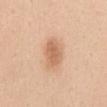Imaged during a routine full-body skin examination; the lesion was not biopsied and no histopathology is available. The subject is a female in their mid- to late 40s. A 15 mm close-up tile from a total-body photography series done for melanoma screening. Longest diameter approximately 4 mm. The lesion-visualizer software estimated an average lesion color of about L≈65 a*≈21 b*≈34 (CIELAB), about 10 CIELAB-L* units darker than the surrounding skin, and a normalized lesion–skin contrast near 6.5. It also reported an automated nevus-likeness rating near 90 out of 100 and lesion-presence confidence of about 100/100. The lesion is located on the abdomen.